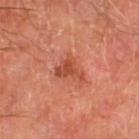Q: Is there a histopathology result?
A: total-body-photography surveillance lesion; no biopsy
Q: Patient demographics?
A: male, approximately 70 years of age
Q: How was this image acquired?
A: total-body-photography crop, ~15 mm field of view
Q: Where on the body is the lesion?
A: the left thigh